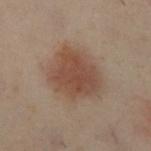biopsy status: total-body-photography surveillance lesion; no biopsy
body site: the left leg
patient: female, aged approximately 60
image source: ~15 mm tile from a whole-body skin photo
image-analysis metrics: an automated nevus-likeness rating near 100 out of 100